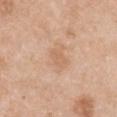Imaged with white-light lighting.
The lesion-visualizer software estimated a lesion area of about 4 mm² and a symmetry-axis asymmetry near 0.25. It also reported a lesion color around L≈64 a*≈19 b*≈34 in CIELAB and roughly 6 lightness units darker than nearby skin. It also reported a border-irregularity rating of about 2.5/10, a color-variation rating of about 1.5/10, and radial color variation of about 0.5. And it measured a nevus-likeness score of about 0/100 and a detector confidence of about 100 out of 100 that the crop contains a lesion.
Approximately 2.5 mm at its widest.
The lesion is on the chest.
The patient is a female in their 60s.
A 15 mm close-up extracted from a 3D total-body photography capture.